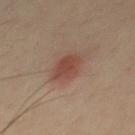| key | value |
|---|---|
| biopsy status | catalogued during a skin exam; not biopsied |
| image source | ~15 mm tile from a whole-body skin photo |
| lesion size | ≈3.5 mm |
| subject | male, in their 40s |
| site | the front of the torso |
| tile lighting | cross-polarized illumination |